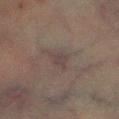Case summary:
– follow-up — imaged on a skin check; not biopsied
– diameter — ≈3 mm
– acquisition — 15 mm crop, total-body photography
– subject — male, aged approximately 70
– lighting — cross-polarized
– body site — the left lower leg